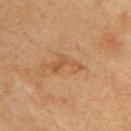Notes:
* notes · total-body-photography surveillance lesion; no biopsy
* subject · male, approximately 70 years of age
* imaging modality · ~15 mm crop, total-body skin-cancer survey
* site · the upper back
* illumination · cross-polarized illumination